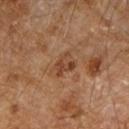patient = male, about 85 years old or older
anatomic site = the right forearm
image = ~15 mm tile from a whole-body skin photo
size = about 3 mm
illumination = cross-polarized illumination